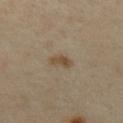The lesion was tiled from a total-body skin photograph and was not biopsied.
The lesion is on the upper back.
A female patient, about 35 years old.
A 15 mm close-up tile from a total-body photography series done for melanoma screening.
Imaged with cross-polarized lighting.